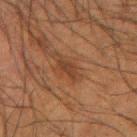Clinical impression: No biopsy was performed on this lesion — it was imaged during a full skin examination and was not determined to be concerning. Image and clinical context: The lesion is on the right forearm. Longest diameter approximately 3 mm. The patient is a male aged 48–52. Cropped from a whole-body photographic skin survey; the tile spans about 15 mm.